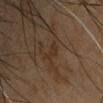No biopsy was performed on this lesion — it was imaged during a full skin examination and was not determined to be concerning.
The lesion is on the chest.
This is a cross-polarized tile.
The lesion-visualizer software estimated an eccentricity of roughly 0.9. And it measured a mean CIELAB color near L≈29 a*≈15 b*≈26, roughly 5 lightness units darker than nearby skin, and a normalized border contrast of about 5.5. It also reported a border-irregularity index near 5.5/10 and peripheral color asymmetry of about 0. It also reported lesion-presence confidence of about 90/100.
A region of skin cropped from a whole-body photographic capture, roughly 15 mm wide.
A male patient, aged 43 to 47.